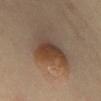Imaged during a routine full-body skin examination; the lesion was not biopsied and no histopathology is available. A roughly 15 mm field-of-view crop from a total-body skin photograph. The lesion is on the mid back. The patient is a female aged 38–42. An algorithmic analysis of the crop reported a footprint of about 14 mm² and an outline eccentricity of about 0.6 (0 = round, 1 = elongated). The analysis additionally found a mean CIELAB color near L≈41 a*≈16 b*≈27, roughly 10 lightness units darker than nearby skin, and a lesion-to-skin contrast of about 8.5 (normalized; higher = more distinct). And it measured a border-irregularity rating of about 4.5/10, a within-lesion color-variation index near 7.5/10, and a peripheral color-asymmetry measure near 2.5. Measured at roughly 5.5 mm in maximum diameter.